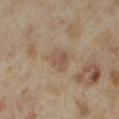No biopsy was performed on this lesion — it was imaged during a full skin examination and was not determined to be concerning.
On the right thigh.
A female patient, in their mid- to late 30s.
The lesion's longest dimension is about 3 mm.
A 15 mm crop from a total-body photograph taken for skin-cancer surveillance.
The tile uses cross-polarized illumination.